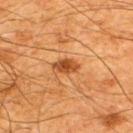The lesion was tiled from a total-body skin photograph and was not biopsied. A male patient about 65 years old. A 15 mm close-up tile from a total-body photography series done for melanoma screening. The lesion's longest dimension is about 3 mm. The lesion is located on the upper back.